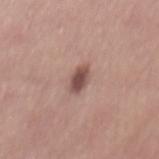| field | value |
|---|---|
| notes | total-body-photography surveillance lesion; no biopsy |
| subject | male, aged 38 to 42 |
| lesion diameter | ≈3 mm |
| image source | 15 mm crop, total-body photography |
| location | the mid back |
| automated metrics | a footprint of about 4 mm² and an eccentricity of roughly 0.85; a lesion color around L≈49 a*≈20 b*≈22 in CIELAB, about 14 CIELAB-L* units darker than the surrounding skin, and a lesion-to-skin contrast of about 9.5 (normalized; higher = more distinct) |